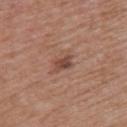Impression: The lesion was photographed on a routine skin check and not biopsied; there is no pathology result. Acquisition and patient details: The patient is a female roughly 50 years of age. The lesion is located on the upper back. The recorded lesion diameter is about 2.5 mm. A 15 mm close-up tile from a total-body photography series done for melanoma screening. Imaged with white-light lighting.